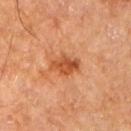Notes:
• biopsy status — imaged on a skin check; not biopsied
• lighting — cross-polarized illumination
• lesion diameter — ~3.5 mm (longest diameter)
• image-analysis metrics — a lesion area of about 6.5 mm², a shape eccentricity near 0.7, and a shape-asymmetry score of about 0.25 (0 = symmetric); a classifier nevus-likeness of about 45/100 and lesion-presence confidence of about 100/100
• site — the arm
• patient — male, about 65 years old
• image source — 15 mm crop, total-body photography